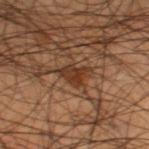{"biopsy_status": "not biopsied; imaged during a skin examination", "image": {"source": "total-body photography crop", "field_of_view_mm": 15}, "lighting": "cross-polarized", "automated_metrics": {"area_mm2_approx": 5.5, "eccentricity": 0.8, "shape_asymmetry": 0.45, "nevus_likeness_0_100": 95, "lesion_detection_confidence_0_100": 65}, "site": "leg", "patient": {"sex": "male", "age_approx": 45}}